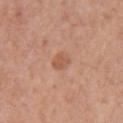The lesion is on the left upper arm. A female patient aged 58 to 62. Cropped from a total-body skin-imaging series; the visible field is about 15 mm.